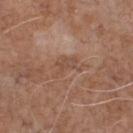Recorded during total-body skin imaging; not selected for excision or biopsy.
The lesion-visualizer software estimated a footprint of about 6 mm² and a shape-asymmetry score of about 0.45 (0 = symmetric). The software also gave a lesion color around L≈49 a*≈19 b*≈28 in CIELAB. And it measured a border-irregularity index near 6/10, internal color variation of about 2 on a 0–10 scale, and peripheral color asymmetry of about 0.5. The software also gave an automated nevus-likeness rating near 0 out of 100 and a detector confidence of about 100 out of 100 that the crop contains a lesion.
On the chest.
A male subject about 70 years old.
Captured under white-light illumination.
A 15 mm close-up extracted from a 3D total-body photography capture.